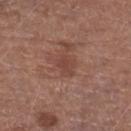workup: total-body-photography surveillance lesion; no biopsy
subject: male, aged approximately 75
location: the leg
lesion size: ≈2.5 mm
imaging modality: 15 mm crop, total-body photography
automated lesion analysis: an average lesion color of about L≈43 a*≈22 b*≈25 (CIELAB), a lesion–skin lightness drop of about 6, and a lesion-to-skin contrast of about 5 (normalized; higher = more distinct); a border-irregularity rating of about 3/10, internal color variation of about 0.5 on a 0–10 scale, and peripheral color asymmetry of about 0; an automated nevus-likeness rating near 0 out of 100 and lesion-presence confidence of about 100/100
illumination: white-light illumination